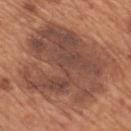Part of a total-body skin-imaging series; this lesion was reviewed on a skin check and was not flagged for biopsy. A lesion tile, about 15 mm wide, cut from a 3D total-body photograph. The total-body-photography lesion software estimated an area of roughly 70 mm², a shape eccentricity near 0.65, and a symmetry-axis asymmetry near 0.3. The software also gave a mean CIELAB color near L≈47 a*≈22 b*≈27 and a normalized border contrast of about 9. It also reported border irregularity of about 6 on a 0–10 scale. The recorded lesion diameter is about 11.5 mm. The lesion is located on the mid back. Imaged with white-light lighting. The subject is a male approximately 65 years of age.